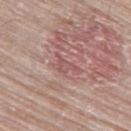Clinical impression:
No biopsy was performed on this lesion — it was imaged during a full skin examination and was not determined to be concerning.
Background:
On the left thigh. The subject is a male aged 78–82. The tile uses white-light illumination. Automated tile analysis of the lesion measured a border-irregularity rating of about 5/10 and a peripheral color-asymmetry measure near 0.5. The software also gave an automated nevus-likeness rating near 0 out of 100 and a detector confidence of about 50 out of 100 that the crop contains a lesion. Cropped from a whole-body photographic skin survey; the tile spans about 15 mm. About 2.5 mm across.